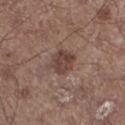Notes:
– notes: imaged on a skin check; not biopsied
– lighting: white-light illumination
– patient: male, aged around 60
– diameter: ≈3.5 mm
– image: ~15 mm crop, total-body skin-cancer survey
– TBP lesion metrics: an area of roughly 7.5 mm²; an automated nevus-likeness rating near 0 out of 100 and lesion-presence confidence of about 100/100
– anatomic site: the leg